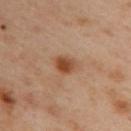This lesion was catalogued during total-body skin photography and was not selected for biopsy. This is a cross-polarized tile. An algorithmic analysis of the crop reported a lesion area of about 4.5 mm², a shape eccentricity near 0.6, and a shape-asymmetry score of about 0.15 (0 = symmetric). The software also gave a lesion color around L≈48 a*≈23 b*≈35 in CIELAB, roughly 12 lightness units darker than nearby skin, and a lesion-to-skin contrast of about 9.5 (normalized; higher = more distinct). It also reported internal color variation of about 4.5 on a 0–10 scale and a peripheral color-asymmetry measure near 1.5. On the upper back. Approximately 2.5 mm at its widest. A female patient roughly 40 years of age. This image is a 15 mm lesion crop taken from a total-body photograph.